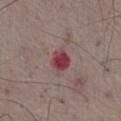The lesion was photographed on a routine skin check and not biopsied; there is no pathology result.
A region of skin cropped from a whole-body photographic capture, roughly 15 mm wide.
A male patient, in their mid- to late 70s.
From the right thigh.